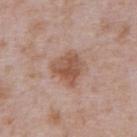| key | value |
|---|---|
| biopsy status | total-body-photography surveillance lesion; no biopsy |
| image source | ~15 mm crop, total-body skin-cancer survey |
| anatomic site | the abdomen |
| lesion size | about 4 mm |
| subject | male, aged 63–67 |
| tile lighting | white-light illumination |